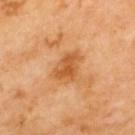Notes:
* notes: imaged on a skin check; not biopsied
* lighting: cross-polarized
* anatomic site: the upper back
* subject: male, approximately 70 years of age
* image: ~15 mm tile from a whole-body skin photo